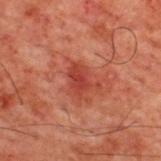Impression:
No biopsy was performed on this lesion — it was imaged during a full skin examination and was not determined to be concerning.
Clinical summary:
Automated tile analysis of the lesion measured an area of roughly 7.5 mm², a shape eccentricity near 0.65, and a symmetry-axis asymmetry near 0.3. The software also gave border irregularity of about 4 on a 0–10 scale and internal color variation of about 2.5 on a 0–10 scale. The analysis additionally found a nevus-likeness score of about 0/100 and a lesion-detection confidence of about 100/100. A 15 mm close-up extracted from a 3D total-body photography capture. Imaged with cross-polarized lighting. The lesion is on the upper back. A male patient, aged 58 to 62.